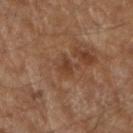Captured during whole-body skin photography for melanoma surveillance; the lesion was not biopsied. The lesion-visualizer software estimated a lesion color around L≈37 a*≈20 b*≈28 in CIELAB, roughly 7 lightness units darker than nearby skin, and a normalized lesion–skin contrast near 6. And it measured radial color variation of about 0.5. And it measured a detector confidence of about 100 out of 100 that the crop contains a lesion. This is a cross-polarized tile. A male patient aged approximately 60. Cropped from a total-body skin-imaging series; the visible field is about 15 mm. The lesion is located on the arm.